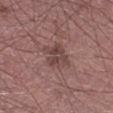Assessment:
Imaged during a routine full-body skin examination; the lesion was not biopsied and no histopathology is available.
Acquisition and patient details:
Captured under white-light illumination. The recorded lesion diameter is about 3.5 mm. A 15 mm crop from a total-body photograph taken for skin-cancer surveillance. A male subject, in their 60s. On the right lower leg.